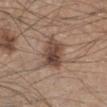| key | value |
|---|---|
| workup | imaged on a skin check; not biopsied |
| image source | ~15 mm crop, total-body skin-cancer survey |
| body site | the right lower leg |
| lighting | white-light illumination |
| subject | male, roughly 45 years of age |